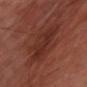Findings:
• notes · imaged on a skin check; not biopsied
• anatomic site · the chest
• patient · male, aged 63–67
• tile lighting · cross-polarized illumination
• image source · ~15 mm crop, total-body skin-cancer survey
• diameter · ~5.5 mm (longest diameter)
• automated lesion analysis · a lesion area of about 18 mm², an outline eccentricity of about 0.65 (0 = round, 1 = elongated), and two-axis asymmetry of about 0.3